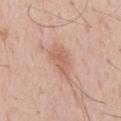biopsy status: total-body-photography surveillance lesion; no biopsy | subject: male, roughly 55 years of age | body site: the mid back | image: total-body-photography crop, ~15 mm field of view.